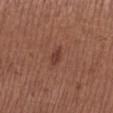notes: imaged on a skin check; not biopsied
patient: female, aged approximately 55
tile lighting: white-light illumination
image source: ~15 mm crop, total-body skin-cancer survey
automated lesion analysis: a footprint of about 2.5 mm², a shape eccentricity near 0.85, and a shape-asymmetry score of about 0.25 (0 = symmetric); a border-irregularity rating of about 2.5/10
body site: the left lower leg